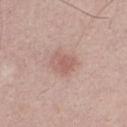Captured during whole-body skin photography for melanoma surveillance; the lesion was not biopsied.
The tile uses white-light illumination.
Automated tile analysis of the lesion measured an area of roughly 6 mm², a shape eccentricity near 0.6, and a shape-asymmetry score of about 0.2 (0 = symmetric). It also reported a border-irregularity rating of about 2/10, internal color variation of about 3 on a 0–10 scale, and peripheral color asymmetry of about 1.
The lesion's longest dimension is about 3 mm.
A roughly 15 mm field-of-view crop from a total-body skin photograph.
A male subject, roughly 30 years of age.